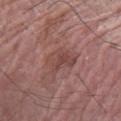workup: no biopsy performed (imaged during a skin exam)
site: the arm
lesion diameter: ~4 mm (longest diameter)
image: total-body-photography crop, ~15 mm field of view
patient: male, about 65 years old
illumination: white-light